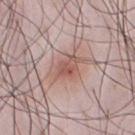– biopsy status · imaged on a skin check; not biopsied
– body site · the chest
– automated lesion analysis · border irregularity of about 4 on a 0–10 scale, a color-variation rating of about 4.5/10, and radial color variation of about 1; a classifier nevus-likeness of about 90/100
– subject · male, approximately 35 years of age
– lesion diameter · about 5 mm
– image · ~15 mm crop, total-body skin-cancer survey